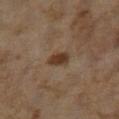notes: imaged on a skin check; not biopsied
diameter: about 2.5 mm
patient: female, in their 60s
illumination: cross-polarized illumination
acquisition: total-body-photography crop, ~15 mm field of view
site: the left lower leg
automated lesion analysis: a lesion area of about 4 mm² and a shape-asymmetry score of about 0.25 (0 = symmetric); a mean CIELAB color near L≈31 a*≈14 b*≈25 and a lesion-to-skin contrast of about 10 (normalized; higher = more distinct); a classifier nevus-likeness of about 95/100 and a lesion-detection confidence of about 100/100